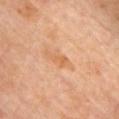Impression: The lesion was photographed on a routine skin check and not biopsied; there is no pathology result. Background: The tile uses cross-polarized illumination. A lesion tile, about 15 mm wide, cut from a 3D total-body photograph. The lesion's longest dimension is about 2.5 mm. A female subject, aged around 65. Automated image analysis of the tile measured an outline eccentricity of about 0.85 (0 = round, 1 = elongated) and a symmetry-axis asymmetry near 0.3. The analysis additionally found a within-lesion color-variation index near 0.5/10 and peripheral color asymmetry of about 0. Located on the chest.